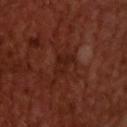* notes · total-body-photography surveillance lesion; no biopsy
* image · ~15 mm tile from a whole-body skin photo
* diameter · ~3 mm (longest diameter)
* patient · male, in their 50s
* lighting · cross-polarized
* location · the upper back